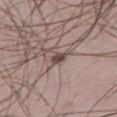biopsy_status: not biopsied; imaged during a skin examination
patient:
  sex: male
  age_approx: 50
image:
  source: total-body photography crop
  field_of_view_mm: 15
lighting: white-light
site: right thigh